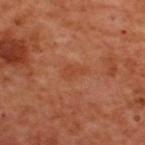<case>
<biopsy_status>not biopsied; imaged during a skin examination</biopsy_status>
</case>